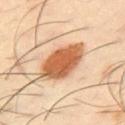Clinical impression:
Recorded during total-body skin imaging; not selected for excision or biopsy.
Background:
The recorded lesion diameter is about 6.5 mm. On the chest. A lesion tile, about 15 mm wide, cut from a 3D total-body photograph. A male subject aged approximately 40. Captured under cross-polarized illumination.